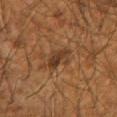Assessment: The lesion was tiled from a total-body skin photograph and was not biopsied. Clinical summary: From the right forearm. The patient is a male in their mid- to late 60s. A 15 mm crop from a total-body photograph taken for skin-cancer surveillance.